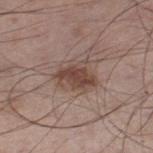Assessment: Part of a total-body skin-imaging series; this lesion was reviewed on a skin check and was not flagged for biopsy. Acquisition and patient details: The subject is a male approximately 60 years of age. A roughly 15 mm field-of-view crop from a total-body skin photograph. The lesion-visualizer software estimated a shape-asymmetry score of about 0.35 (0 = symmetric). And it measured a lesion color around L≈45 a*≈18 b*≈24 in CIELAB, roughly 11 lightness units darker than nearby skin, and a normalized lesion–skin contrast near 8.5. The analysis additionally found internal color variation of about 2.5 on a 0–10 scale and a peripheral color-asymmetry measure near 1. Located on the left thigh. Imaged with white-light lighting.